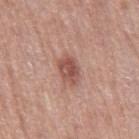{
  "biopsy_status": "not biopsied; imaged during a skin examination"
}